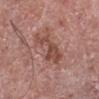| feature | finding |
|---|---|
| imaging modality | total-body-photography crop, ~15 mm field of view |
| patient | male, roughly 75 years of age |
| diameter | ≈5 mm |
| tile lighting | white-light illumination |
| automated lesion analysis | a lesion area of about 9.5 mm², an eccentricity of roughly 0.85, and a shape-asymmetry score of about 0.5 (0 = symmetric); border irregularity of about 6.5 on a 0–10 scale and a within-lesion color-variation index near 2.5/10; an automated nevus-likeness rating near 0 out of 100 and a lesion-detection confidence of about 100/100 |
| site | the left lower leg |